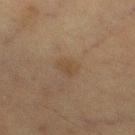Context:
A female subject, approximately 55 years of age. Cropped from a whole-body photographic skin survey; the tile spans about 15 mm. The lesion is on the right thigh.